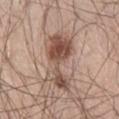<lesion>
<biopsy_status>not biopsied; imaged during a skin examination</biopsy_status>
<lesion_size>
  <long_diameter_mm_approx>7.0</long_diameter_mm_approx>
</lesion_size>
<patient>
  <sex>male</sex>
  <age_approx>40</age_approx>
</patient>
<site>right thigh</site>
<lighting>white-light</lighting>
<image>
  <source>total-body photography crop</source>
  <field_of_view_mm>15</field_of_view_mm>
</image>
</lesion>